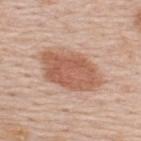Impression: Imaged during a routine full-body skin examination; the lesion was not biopsied and no histopathology is available. Background: From the upper back. The tile uses white-light illumination. The lesion's longest dimension is about 7.5 mm. The subject is a male in their 60s. A lesion tile, about 15 mm wide, cut from a 3D total-body photograph.